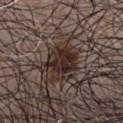This lesion was catalogued during total-body skin photography and was not selected for biopsy.
A male patient aged 48 to 52.
Captured under white-light illumination.
The lesion's longest dimension is about 5 mm.
A lesion tile, about 15 mm wide, cut from a 3D total-body photograph.
The lesion is located on the chest.